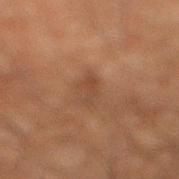Imaged during a routine full-body skin examination; the lesion was not biopsied and no histopathology is available. Imaged with cross-polarized lighting. The lesion is on the right lower leg. Cropped from a whole-body photographic skin survey; the tile spans about 15 mm. About 3.5 mm across. The subject is a male aged 63–67.